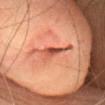Recorded during total-body skin imaging; not selected for excision or biopsy. About 3 mm across. From the head or neck. Cropped from a whole-body photographic skin survey; the tile spans about 15 mm. The patient is a female about 60 years old. The lesion-visualizer software estimated an average lesion color of about L≈47 a*≈29 b*≈32 (CIELAB) and a lesion-to-skin contrast of about 9 (normalized; higher = more distinct). The software also gave an automated nevus-likeness rating near 0 out of 100 and lesion-presence confidence of about 55/100.